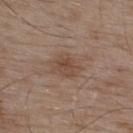{"biopsy_status": "not biopsied; imaged during a skin examination", "patient": {"sex": "male", "age_approx": 55}, "lesion_size": {"long_diameter_mm_approx": 3.5}, "image": {"source": "total-body photography crop", "field_of_view_mm": 15}, "lighting": "white-light", "site": "upper back"}